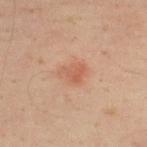The lesion is located on the upper back. This is a cross-polarized tile. The patient is a male roughly 50 years of age. A region of skin cropped from a whole-body photographic capture, roughly 15 mm wide. Longest diameter approximately 3 mm. An algorithmic analysis of the crop reported an eccentricity of roughly 0.65 and a shape-asymmetry score of about 0.3 (0 = symmetric). And it measured a mean CIELAB color near L≈52 a*≈22 b*≈29, a lesion–skin lightness drop of about 7, and a lesion-to-skin contrast of about 5.5 (normalized; higher = more distinct).